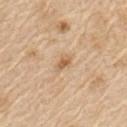workup = imaged on a skin check; not biopsied | location = the left upper arm | imaging modality = ~15 mm crop, total-body skin-cancer survey | diameter = ~3.5 mm (longest diameter) | TBP lesion metrics = a border-irregularity index near 3/10; a nevus-likeness score of about 15/100 and a detector confidence of about 100 out of 100 that the crop contains a lesion | illumination = white-light | patient = male, roughly 70 years of age.